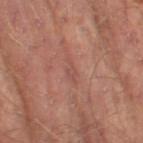Context: A 15 mm close-up tile from a total-body photography series done for melanoma screening. Automated image analysis of the tile measured an average lesion color of about L≈48 a*≈23 b*≈24 (CIELAB), about 6 CIELAB-L* units darker than the surrounding skin, and a normalized border contrast of about 4.5. And it measured a color-variation rating of about 0/10 and peripheral color asymmetry of about 0. On the leg. Imaged with cross-polarized lighting. The patient is a male aged 63 to 67.